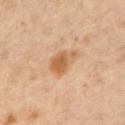The lesion was photographed on a routine skin check and not biopsied; there is no pathology result.
A close-up tile cropped from a whole-body skin photograph, about 15 mm across.
Located on the left upper arm.
The subject is a male aged approximately 55.